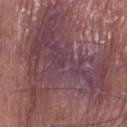Q: Was this lesion biopsied?
A: no biopsy performed (imaged during a skin exam)
Q: Lesion location?
A: the right thigh
Q: Automated lesion metrics?
A: an automated nevus-likeness rating near 0 out of 100 and lesion-presence confidence of about 65/100
Q: What are the patient's age and sex?
A: male, about 85 years old or older
Q: How was this image acquired?
A: ~15 mm crop, total-body skin-cancer survey
Q: Illumination type?
A: white-light
Q: Lesion size?
A: ~16.5 mm (longest diameter)